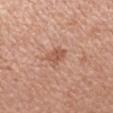Q: Where on the body is the lesion?
A: the left forearm
Q: Patient demographics?
A: female, roughly 35 years of age
Q: What kind of image is this?
A: ~15 mm tile from a whole-body skin photo